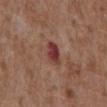This lesion was catalogued during total-body skin photography and was not selected for biopsy. A male subject aged 73 to 77. Captured under white-light illumination. A 15 mm close-up extracted from a 3D total-body photography capture. The lesion is on the abdomen.